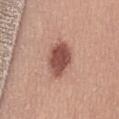- workup: total-body-photography surveillance lesion; no biopsy
- image: 15 mm crop, total-body photography
- TBP lesion metrics: an area of roughly 11 mm², an outline eccentricity of about 0.75 (0 = round, 1 = elongated), and a symmetry-axis asymmetry near 0.2; border irregularity of about 1.5 on a 0–10 scale, a color-variation rating of about 3/10, and peripheral color asymmetry of about 1; an automated nevus-likeness rating near 90 out of 100 and a lesion-detection confidence of about 100/100
- body site: the abdomen
- lesion size: ≈4.5 mm
- lighting: white-light
- patient: female, aged approximately 35